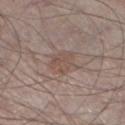Impression:
Captured during whole-body skin photography for melanoma surveillance; the lesion was not biopsied.
Background:
A close-up tile cropped from a whole-body skin photograph, about 15 mm across. The subject is a male in their 60s. Captured under white-light illumination. Located on the left lower leg.